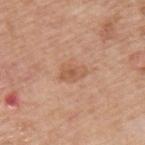- biopsy status — imaged on a skin check; not biopsied
- patient — male, aged 73–77
- imaging modality — total-body-photography crop, ~15 mm field of view
- location — the left upper arm
- tile lighting — white-light illumination